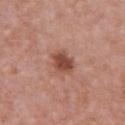Case summary:
* biopsy status — catalogued during a skin exam; not biopsied
* location — the chest
* subject — female, aged 38 to 42
* diameter — ~3.5 mm (longest diameter)
* image source — ~15 mm tile from a whole-body skin photo
* tile lighting — white-light illumination